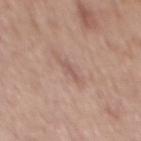Notes:
* biopsy status — total-body-photography surveillance lesion; no biopsy
* automated lesion analysis — a footprint of about 2 mm², an outline eccentricity of about 0.95 (0 = round, 1 = elongated), and two-axis asymmetry of about 0.4; a mean CIELAB color near L≈56 a*≈19 b*≈24, a lesion–skin lightness drop of about 7, and a normalized lesion–skin contrast near 5.5; a border-irregularity rating of about 4.5/10, internal color variation of about 0 on a 0–10 scale, and peripheral color asymmetry of about 0; an automated nevus-likeness rating near 0 out of 100 and a lesion-detection confidence of about 100/100
* image — 15 mm crop, total-body photography
* site — the mid back
* subject — male, aged approximately 70